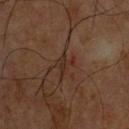This image is a 15 mm lesion crop taken from a total-body photograph. On the chest. The patient is a male roughly 60 years of age.